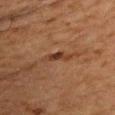{
  "biopsy_status": "not biopsied; imaged during a skin examination",
  "image": {
    "source": "total-body photography crop",
    "field_of_view_mm": 15
  },
  "patient": {
    "sex": "male",
    "age_approx": 45
  },
  "automated_metrics": {
    "area_mm2_approx": 4.0,
    "eccentricity": 0.9,
    "shape_asymmetry": 0.3,
    "cielab_L": 31,
    "cielab_a": 18,
    "cielab_b": 27,
    "vs_skin_darker_L": 7.0,
    "vs_skin_contrast_norm": 7.5,
    "nevus_likeness_0_100": 70,
    "lesion_detection_confidence_0_100": 100
  },
  "lesion_size": {
    "long_diameter_mm_approx": 3.0
  },
  "site": "front of the torso",
  "lighting": "cross-polarized"
}